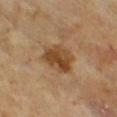Notes:
• subject: female, approximately 60 years of age
• image: ~15 mm crop, total-body skin-cancer survey
• automated metrics: two-axis asymmetry of about 0.2; an average lesion color of about L≈41 a*≈17 b*≈33 (CIELAB), roughly 10 lightness units darker than nearby skin, and a lesion-to-skin contrast of about 9 (normalized; higher = more distinct); a nevus-likeness score of about 70/100
• tile lighting: cross-polarized
• site: the front of the torso